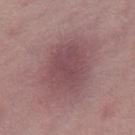Impression:
The lesion was photographed on a routine skin check and not biopsied; there is no pathology result.
Clinical summary:
The lesion is on the right thigh. Cropped from a total-body skin-imaging series; the visible field is about 15 mm. Captured under white-light illumination. About 5.5 mm across. A female subject approximately 45 years of age.